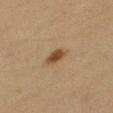Background:
Cropped from a total-body skin-imaging series; the visible field is about 15 mm. Longest diameter approximately 3 mm. Located on the right thigh. A female subject about 55 years old. Captured under cross-polarized illumination.